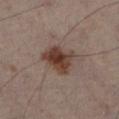The lesion was photographed on a routine skin check and not biopsied; there is no pathology result.
The lesion's longest dimension is about 4.5 mm.
The lesion is located on the left leg.
Cropped from a total-body skin-imaging series; the visible field is about 15 mm.
A male subject about 50 years old.